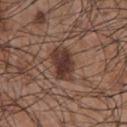Q: What is the imaging modality?
A: 15 mm crop, total-body photography
Q: What is the lesion's diameter?
A: about 4 mm
Q: What lighting was used for the tile?
A: white-light illumination
Q: Where on the body is the lesion?
A: the chest
Q: Patient demographics?
A: male, about 55 years old